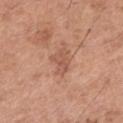Findings:
– workup · catalogued during a skin exam; not biopsied
– imaging modality · ~15 mm crop, total-body skin-cancer survey
– subject · male, approximately 65 years of age
– location · the left thigh
– illumination · white-light illumination
– automated metrics · a lesion color around L≈55 a*≈23 b*≈30 in CIELAB and a lesion–skin lightness drop of about 8; internal color variation of about 3 on a 0–10 scale and radial color variation of about 1; a lesion-detection confidence of about 100/100